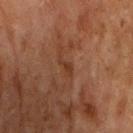workup: total-body-photography surveillance lesion; no biopsy
subject: female, approximately 60 years of age
location: the right upper arm
imaging modality: ~15 mm crop, total-body skin-cancer survey
diameter: ≈3 mm
automated metrics: a shape eccentricity near 0.9 and a shape-asymmetry score of about 0.4 (0 = symmetric); a border-irregularity rating of about 4.5/10, internal color variation of about 0 on a 0–10 scale, and a peripheral color-asymmetry measure near 0